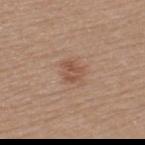<tbp_lesion>
<biopsy_status>not biopsied; imaged during a skin examination</biopsy_status>
<automated_metrics>
  <area_mm2_approx>4.5</area_mm2_approx>
  <eccentricity>0.55</eccentricity>
  <cielab_L>51</cielab_L>
  <cielab_a>21</cielab_a>
  <cielab_b>29</cielab_b>
  <lesion_detection_confidence_0_100>100</lesion_detection_confidence_0_100>
</automated_metrics>
<patient>
  <sex>male</sex>
  <age_approx>40</age_approx>
</patient>
<lighting>white-light</lighting>
<image>
  <source>total-body photography crop</source>
  <field_of_view_mm>15</field_of_view_mm>
</image>
<site>back</site>
<lesion_size>
  <long_diameter_mm_approx>2.5</long_diameter_mm_approx>
</lesion_size>
</tbp_lesion>